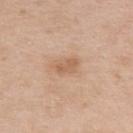Assessment: The lesion was tiled from a total-body skin photograph and was not biopsied. Image and clinical context: A female patient aged around 40. The lesion-visualizer software estimated a mean CIELAB color near L≈61 a*≈19 b*≈33 and about 8 CIELAB-L* units darker than the surrounding skin. And it measured border irregularity of about 2.5 on a 0–10 scale, internal color variation of about 2 on a 0–10 scale, and peripheral color asymmetry of about 0.5. Captured under white-light illumination. A 15 mm close-up extracted from a 3D total-body photography capture. On the left upper arm. The recorded lesion diameter is about 3.5 mm.